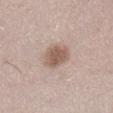Background: The patient is a female about 40 years old. The lesion-visualizer software estimated an average lesion color of about L≈57 a*≈17 b*≈24 (CIELAB), roughly 12 lightness units darker than nearby skin, and a lesion-to-skin contrast of about 8 (normalized; higher = more distinct). The software also gave an automated nevus-likeness rating near 75 out of 100 and lesion-presence confidence of about 100/100. This image is a 15 mm lesion crop taken from a total-body photograph. From the leg.